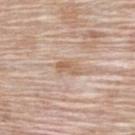Part of a total-body skin-imaging series; this lesion was reviewed on a skin check and was not flagged for biopsy.
A female subject, aged 63–67.
This is a white-light tile.
From the back.
Longest diameter approximately 3 mm.
Cropped from a whole-body photographic skin survey; the tile spans about 15 mm.